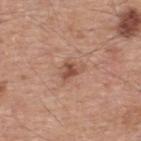Captured during whole-body skin photography for melanoma surveillance; the lesion was not biopsied. A 15 mm crop from a total-body photograph taken for skin-cancer surveillance. The total-body-photography lesion software estimated an area of roughly 4 mm² and a shape-asymmetry score of about 0.4 (0 = symmetric). The analysis additionally found a nevus-likeness score of about 50/100. The lesion is located on the back. Longest diameter approximately 3 mm. A male patient, in their mid-50s.